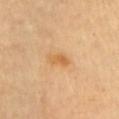• workup · catalogued during a skin exam; not biopsied
• size · about 2.5 mm
• imaging modality · total-body-photography crop, ~15 mm field of view
• body site · the left upper arm
• tile lighting · cross-polarized illumination
• patient · aged around 60